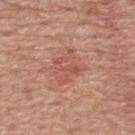workup=no biopsy performed (imaged during a skin exam) | location=the back | automated metrics=a lesion area of about 8.5 mm² and an eccentricity of roughly 0.6; a mean CIELAB color near L≈54 a*≈26 b*≈29 and a normalized border contrast of about 5 | lesion diameter=~4 mm (longest diameter) | image source=~15 mm tile from a whole-body skin photo | subject=male, aged 78 to 82.